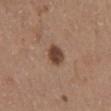subject = male, about 55 years old
location = the back
image-analysis metrics = a shape eccentricity near 0.6 and two-axis asymmetry of about 0.15; a classifier nevus-likeness of about 90/100 and lesion-presence confidence of about 100/100
image = ~15 mm tile from a whole-body skin photo